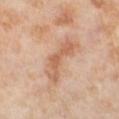No biopsy was performed on this lesion — it was imaged during a full skin examination and was not determined to be concerning. A female patient approximately 55 years of age. About 5.5 mm across. From the left lower leg. Imaged with cross-polarized lighting. Automated tile analysis of the lesion measured an area of roughly 10 mm², an outline eccentricity of about 0.9 (0 = round, 1 = elongated), and two-axis asymmetry of about 0.5. The software also gave an average lesion color of about L≈62 a*≈22 b*≈34 (CIELAB). A 15 mm close-up tile from a total-body photography series done for melanoma screening.